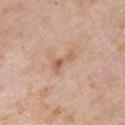Q: Was this lesion biopsied?
A: no biopsy performed (imaged during a skin exam)
Q: Lesion location?
A: the right upper arm
Q: What kind of image is this?
A: total-body-photography crop, ~15 mm field of view
Q: Who is the patient?
A: female, approximately 70 years of age